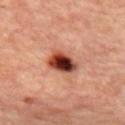Q: Is there a histopathology result?
A: no biopsy performed (imaged during a skin exam)
Q: Where on the body is the lesion?
A: the mid back
Q: How was this image acquired?
A: ~15 mm tile from a whole-body skin photo
Q: What are the patient's age and sex?
A: female, aged 43–47